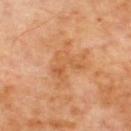The lesion was tiled from a total-body skin photograph and was not biopsied. Measured at roughly 4 mm in maximum diameter. An algorithmic analysis of the crop reported radial color variation of about 1.5. And it measured a nevus-likeness score of about 0/100 and lesion-presence confidence of about 100/100. A 15 mm close-up tile from a total-body photography series done for melanoma screening. From the upper back. The patient is a male roughly 70 years of age.